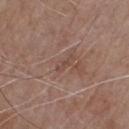notes: no biopsy performed (imaged during a skin exam); anatomic site: the chest; subject: male, aged 78 to 82; lesion size: about 2.5 mm; image source: 15 mm crop, total-body photography; illumination: white-light illumination.